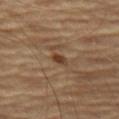This lesion was catalogued during total-body skin photography and was not selected for biopsy. Located on the leg. This image is a 15 mm lesion crop taken from a total-body photograph. A male patient aged approximately 60.